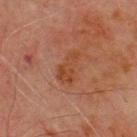Impression:
The lesion was tiled from a total-body skin photograph and was not biopsied.
Image and clinical context:
A male patient aged 68–72. Located on the front of the torso. A close-up tile cropped from a whole-body skin photograph, about 15 mm across.